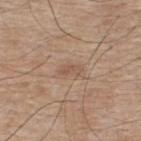Part of a total-body skin-imaging series; this lesion was reviewed on a skin check and was not flagged for biopsy. From the upper back. An algorithmic analysis of the crop reported an average lesion color of about L≈54 a*≈17 b*≈29 (CIELAB). And it measured a nevus-likeness score of about 0/100 and a lesion-detection confidence of about 100/100. The recorded lesion diameter is about 2.5 mm. Cropped from a whole-body photographic skin survey; the tile spans about 15 mm. This is a white-light tile. A male subject, in their mid- to late 70s.